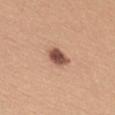Notes:
• TBP lesion metrics · a lesion area of about 5 mm², an outline eccentricity of about 0.75 (0 = round, 1 = elongated), and a shape-asymmetry score of about 0.2 (0 = symmetric); a lesion color around L≈52 a*≈23 b*≈28 in CIELAB and about 17 CIELAB-L* units darker than the surrounding skin; a border-irregularity rating of about 2/10, a color-variation rating of about 3.5/10, and a peripheral color-asymmetry measure near 1
• patient · female, aged approximately 30
• tile lighting · white-light
• lesion size · about 3 mm
• anatomic site · the upper back
• image · total-body-photography crop, ~15 mm field of view